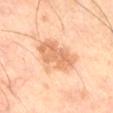Assessment:
Imaged during a routine full-body skin examination; the lesion was not biopsied and no histopathology is available.
Clinical summary:
A 15 mm crop from a total-body photograph taken for skin-cancer surveillance. The lesion is located on the right thigh. Imaged with cross-polarized lighting. The patient is a male about 60 years old. Automated image analysis of the tile measured a lesion–skin lightness drop of about 11 and a lesion-to-skin contrast of about 6.5 (normalized; higher = more distinct). And it measured internal color variation of about 3.5 on a 0–10 scale and radial color variation of about 1. The software also gave a classifier nevus-likeness of about 15/100 and a detector confidence of about 100 out of 100 that the crop contains a lesion. Approximately 5 mm at its widest.